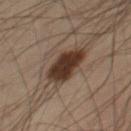Part of a total-body skin-imaging series; this lesion was reviewed on a skin check and was not flagged for biopsy.
A male patient aged approximately 55.
Captured under cross-polarized illumination.
The lesion is on the leg.
Cropped from a total-body skin-imaging series; the visible field is about 15 mm.
Longest diameter approximately 5 mm.